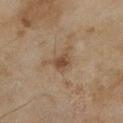Q: Where on the body is the lesion?
A: the leg
Q: Who is the patient?
A: female, aged 58 to 62
Q: What is the imaging modality?
A: 15 mm crop, total-body photography